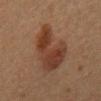- notes: imaged on a skin check; not biopsied
- patient: male, aged 58–62
- body site: the mid back
- image: ~15 mm crop, total-body skin-cancer survey
- illumination: cross-polarized illumination
- lesion diameter: about 7.5 mm